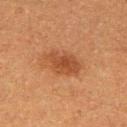Findings:
• follow-up · no biopsy performed (imaged during a skin exam)
• acquisition · 15 mm crop, total-body photography
• illumination · cross-polarized illumination
• patient · female, aged 38 to 42
• lesion diameter · about 4.5 mm
• anatomic site · the right thigh